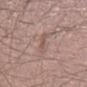{"biopsy_status": "not biopsied; imaged during a skin examination", "image": {"source": "total-body photography crop", "field_of_view_mm": 15}, "patient": {"sex": "male", "age_approx": 25}, "site": "right lower leg", "automated_metrics": {"area_mm2_approx": 3.5, "eccentricity": 0.9, "shape_asymmetry": 0.4, "color_variation_0_10": 1.5, "peripheral_color_asymmetry": 0.0}, "lighting": "white-light"}